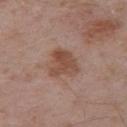Clinical impression:
No biopsy was performed on this lesion — it was imaged during a full skin examination and was not determined to be concerning.
Background:
A 15 mm close-up extracted from a 3D total-body photography capture. Located on the right upper arm. The tile uses white-light illumination. The subject is a male in their mid- to late 50s. Measured at roughly 3.5 mm in maximum diameter.